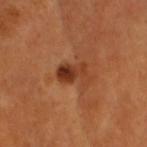Q: Was a biopsy performed?
A: catalogued during a skin exam; not biopsied
Q: Illumination type?
A: cross-polarized
Q: Lesion location?
A: the head or neck
Q: How large is the lesion?
A: ≈3.5 mm
Q: What did automated image analysis measure?
A: a lesion area of about 7 mm² and a symmetry-axis asymmetry near 0.35; an automated nevus-likeness rating near 80 out of 100 and a lesion-detection confidence of about 100/100
Q: What kind of image is this?
A: ~15 mm tile from a whole-body skin photo
Q: Who is the patient?
A: male, aged approximately 60